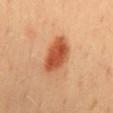Findings:
- workup — catalogued during a skin exam; not biopsied
- acquisition — 15 mm crop, total-body photography
- automated lesion analysis — an outline eccentricity of about 0.8 (0 = round, 1 = elongated) and a shape-asymmetry score of about 0.2 (0 = symmetric); a color-variation rating of about 5/10 and a peripheral color-asymmetry measure near 1.5
- patient — male, in their 40s
- anatomic site — the mid back
- lesion size — ~5 mm (longest diameter)
- tile lighting — cross-polarized illumination